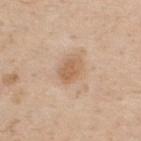This lesion was catalogued during total-body skin photography and was not selected for biopsy. Captured under white-light illumination. Longest diameter approximately 3.5 mm. A male patient, roughly 60 years of age. A roughly 15 mm field-of-view crop from a total-body skin photograph. From the back.